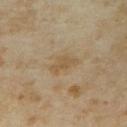Clinical impression: This lesion was catalogued during total-body skin photography and was not selected for biopsy. Context: Imaged with cross-polarized lighting. Located on the right upper arm. A female subject aged around 35. The lesion's longest dimension is about 3.5 mm. A region of skin cropped from a whole-body photographic capture, roughly 15 mm wide.